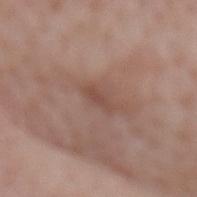Case summary:
* workup: total-body-photography surveillance lesion; no biopsy
* tile lighting: white-light illumination
* location: the back
* image-analysis metrics: a shape eccentricity near 0.9 and two-axis asymmetry of about 0.2; a color-variation rating of about 0.5/10 and a peripheral color-asymmetry measure near 0; a nevus-likeness score of about 0/100 and a detector confidence of about 95 out of 100 that the crop contains a lesion
* subject: female, aged 68–72
* lesion diameter: ≈3 mm
* image: ~15 mm tile from a whole-body skin photo